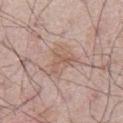Findings:
• biopsy status — no biopsy performed (imaged during a skin exam)
• acquisition — total-body-photography crop, ~15 mm field of view
• tile lighting — white-light
• anatomic site — the abdomen
• patient — male, approximately 65 years of age
• lesion size — ≈3.5 mm